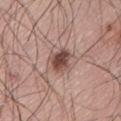Part of a total-body skin-imaging series; this lesion was reviewed on a skin check and was not flagged for biopsy.
The lesion's longest dimension is about 3.5 mm.
The lesion is located on the lower back.
This is a white-light tile.
The subject is a male in their mid- to late 60s.
A 15 mm close-up extracted from a 3D total-body photography capture.
The total-body-photography lesion software estimated a border-irregularity index near 2.5/10, internal color variation of about 4 on a 0–10 scale, and peripheral color asymmetry of about 1.